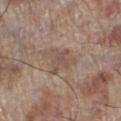biopsy status: imaged on a skin check; not biopsied | illumination: white-light | lesion size: ≈3 mm | acquisition: total-body-photography crop, ~15 mm field of view | image-analysis metrics: a shape eccentricity near 0.75; a lesion-detection confidence of about 100/100 | location: the left lower leg | subject: male, about 70 years old.